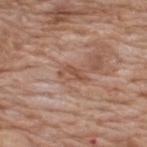<record>
<biopsy_status>not biopsied; imaged during a skin examination</biopsy_status>
<site>upper back</site>
<patient>
  <sex>male</sex>
  <age_approx>60</age_approx>
</patient>
<image>
  <source>total-body photography crop</source>
  <field_of_view_mm>15</field_of_view_mm>
</image>
</record>